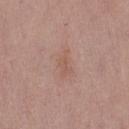Part of a total-body skin-imaging series; this lesion was reviewed on a skin check and was not flagged for biopsy. Captured under white-light illumination. The lesion is on the front of the torso. A roughly 15 mm field-of-view crop from a total-body skin photograph. An algorithmic analysis of the crop reported an outline eccentricity of about 0.85 (0 = round, 1 = elongated) and a symmetry-axis asymmetry near 0.4. It also reported a nevus-likeness score of about 0/100 and a detector confidence of about 100 out of 100 that the crop contains a lesion. The subject is a female in their 50s. Longest diameter approximately 3 mm.